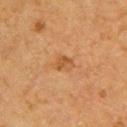location = the upper back
image = total-body-photography crop, ~15 mm field of view
TBP lesion metrics = a footprint of about 4 mm², a shape eccentricity near 0.65, and a symmetry-axis asymmetry near 0.3; border irregularity of about 3 on a 0–10 scale, a within-lesion color-variation index near 2.5/10, and radial color variation of about 0.5; a nevus-likeness score of about 5/100 and a detector confidence of about 100 out of 100 that the crop contains a lesion
illumination = cross-polarized
patient = female, aged around 60
size = ≈2.5 mm